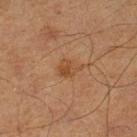Imaged during a routine full-body skin examination; the lesion was not biopsied and no histopathology is available.
A male subject, aged 63 to 67.
The lesion's longest dimension is about 3 mm.
The lesion-visualizer software estimated a footprint of about 5 mm² and a shape eccentricity near 0.7. The analysis additionally found a lesion color around L≈37 a*≈18 b*≈29 in CIELAB, roughly 6 lightness units darker than nearby skin, and a normalized lesion–skin contrast near 6. The analysis additionally found a classifier nevus-likeness of about 10/100.
Located on the right lower leg.
A region of skin cropped from a whole-body photographic capture, roughly 15 mm wide.